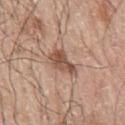Notes:
– follow-up · total-body-photography surveillance lesion; no biopsy
– body site · the left arm
– image source · total-body-photography crop, ~15 mm field of view
– patient · male, about 70 years old
– TBP lesion metrics · a border-irregularity rating of about 3.5/10, a color-variation rating of about 4.5/10, and radial color variation of about 1.5; a nevus-likeness score of about 75/100 and a lesion-detection confidence of about 100/100